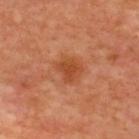Q: Was this lesion biopsied?
A: total-body-photography surveillance lesion; no biopsy
Q: What is the imaging modality?
A: ~15 mm crop, total-body skin-cancer survey
Q: How large is the lesion?
A: ≈2.5 mm
Q: Lesion location?
A: the upper back
Q: Patient demographics?
A: aged 63–67